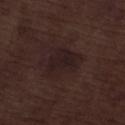Q: Was a biopsy performed?
A: imaged on a skin check; not biopsied
Q: How was this image acquired?
A: total-body-photography crop, ~15 mm field of view
Q: What lighting was used for the tile?
A: white-light illumination
Q: Who is the patient?
A: male, roughly 70 years of age
Q: Where on the body is the lesion?
A: the left lower leg
Q: Automated lesion metrics?
A: an area of roughly 12 mm², a shape eccentricity near 0.7, and a shape-asymmetry score of about 0.3 (0 = symmetric); an automated nevus-likeness rating near 0 out of 100 and a lesion-detection confidence of about 100/100
Q: Lesion size?
A: ~5 mm (longest diameter)